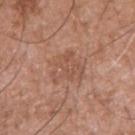Q: How large is the lesion?
A: ~4.5 mm (longest diameter)
Q: Patient demographics?
A: male, in their mid-70s
Q: What kind of image is this?
A: 15 mm crop, total-body photography
Q: Lesion location?
A: the right upper arm